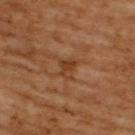This image is a 15 mm lesion crop taken from a total-body photograph.
This is a cross-polarized tile.
On the upper back.
A male subject in their mid-60s.